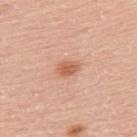anatomic site = the upper back; image = 15 mm crop, total-body photography; patient = male, aged 58 to 62.